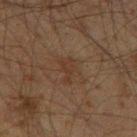Impression: This lesion was catalogued during total-body skin photography and was not selected for biopsy. Acquisition and patient details: Cropped from a whole-body photographic skin survey; the tile spans about 15 mm. On the left thigh. The tile uses cross-polarized illumination. The recorded lesion diameter is about 3.5 mm. The patient is a male in their 60s.